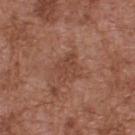| feature | finding |
|---|---|
| biopsy status | imaged on a skin check; not biopsied |
| automated metrics | a mean CIELAB color near L≈44 a*≈22 b*≈29; a border-irregularity index near 3/10, a within-lesion color-variation index near 2/10, and a peripheral color-asymmetry measure near 0.5; a nevus-likeness score of about 0/100 and a lesion-detection confidence of about 100/100 |
| body site | the upper back |
| subject | male, approximately 75 years of age |
| lesion diameter | about 3 mm |
| lighting | white-light illumination |
| image source | 15 mm crop, total-body photography |